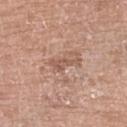| key | value |
|---|---|
| biopsy status | no biopsy performed (imaged during a skin exam) |
| illumination | white-light |
| body site | the right lower leg |
| lesion size | ~3.5 mm (longest diameter) |
| automated metrics | a lesion area of about 3.5 mm², an outline eccentricity of about 0.95 (0 = round, 1 = elongated), and two-axis asymmetry of about 0.5; a lesion color around L≈55 a*≈20 b*≈28 in CIELAB, a lesion–skin lightness drop of about 9, and a lesion-to-skin contrast of about 6.5 (normalized; higher = more distinct); a border-irregularity rating of about 6.5/10 and a within-lesion color-variation index near 1/10; an automated nevus-likeness rating near 0 out of 100 |
| image source | ~15 mm tile from a whole-body skin photo |
| patient | female, about 75 years old |